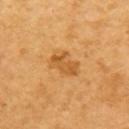Clinical impression: Part of a total-body skin-imaging series; this lesion was reviewed on a skin check and was not flagged for biopsy. Background: A female patient aged around 55. A 15 mm crop from a total-body photograph taken for skin-cancer surveillance. Located on the upper back.